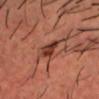Assessment:
Imaged during a routine full-body skin examination; the lesion was not biopsied and no histopathology is available.
Clinical summary:
The lesion is on the head or neck. This image is a 15 mm lesion crop taken from a total-body photograph. The patient is a male aged 33–37. Imaged with cross-polarized lighting.